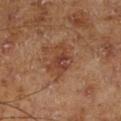notes=no biopsy performed (imaged during a skin exam) | site=the leg | image source=total-body-photography crop, ~15 mm field of view | patient=male, aged approximately 70.